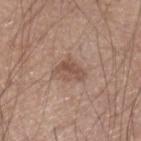Part of a total-body skin-imaging series; this lesion was reviewed on a skin check and was not flagged for biopsy. A 15 mm close-up tile from a total-body photography series done for melanoma screening. Automated tile analysis of the lesion measured a lesion color around L≈51 a*≈18 b*≈26 in CIELAB, roughly 9 lightness units darker than nearby skin, and a normalized lesion–skin contrast near 6.5. The analysis additionally found border irregularity of about 3.5 on a 0–10 scale, internal color variation of about 3.5 on a 0–10 scale, and a peripheral color-asymmetry measure near 1. Longest diameter approximately 3.5 mm. The tile uses white-light illumination. The subject is a male roughly 55 years of age.